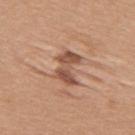notes = no biopsy performed (imaged during a skin exam)
tile lighting = white-light illumination
image = 15 mm crop, total-body photography
anatomic site = the right upper arm
patient = female, in their 20s